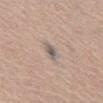<case>
  <biopsy_status>not biopsied; imaged during a skin examination</biopsy_status>
  <site>mid back</site>
  <image>
    <source>total-body photography crop</source>
    <field_of_view_mm>15</field_of_view_mm>
  </image>
  <lighting>white-light</lighting>
  <patient>
    <sex>female</sex>
    <age_approx>80</age_approx>
  </patient>
</case>